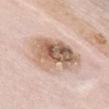Part of a total-body skin-imaging series; this lesion was reviewed on a skin check and was not flagged for biopsy.
Located on the front of the torso.
A close-up tile cropped from a whole-body skin photograph, about 15 mm across.
A female patient, roughly 65 years of age.